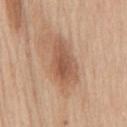| feature | finding |
|---|---|
| biopsy status | total-body-photography surveillance lesion; no biopsy |
| automated lesion analysis | a lesion color around L≈55 a*≈21 b*≈31 in CIELAB, roughly 11 lightness units darker than nearby skin, and a normalized border contrast of about 7 |
| body site | the back |
| patient | male, aged 58–62 |
| lighting | white-light |
| imaging modality | total-body-photography crop, ~15 mm field of view |